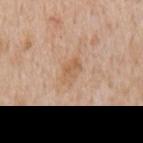The lesion was photographed on a routine skin check and not biopsied; there is no pathology result. A male subject aged 63 to 67. Cropped from a whole-body photographic skin survey; the tile spans about 15 mm. Measured at roughly 3 mm in maximum diameter. This is a white-light tile. Located on the mid back. Automated image analysis of the tile measured a border-irregularity index near 3/10, internal color variation of about 3 on a 0–10 scale, and a peripheral color-asymmetry measure near 1. It also reported a nevus-likeness score of about 0/100 and lesion-presence confidence of about 100/100.